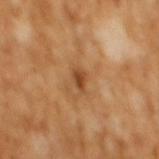Q: Was this lesion biopsied?
A: imaged on a skin check; not biopsied
Q: Automated lesion metrics?
A: border irregularity of about 3 on a 0–10 scale, internal color variation of about 2.5 on a 0–10 scale, and peripheral color asymmetry of about 0.5
Q: Illumination type?
A: cross-polarized illumination
Q: Patient demographics?
A: male, aged around 65
Q: What is the imaging modality?
A: ~15 mm crop, total-body skin-cancer survey
Q: Lesion size?
A: about 3 mm